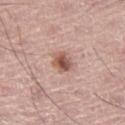  biopsy_status: not biopsied; imaged during a skin examination
  site: left leg
  patient:
    sex: male
    age_approx: 70
  image:
    source: total-body photography crop
    field_of_view_mm: 15